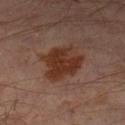follow-up = no biopsy performed (imaged during a skin exam)
tile lighting = cross-polarized
patient = male, aged 63 to 67
size = about 5 mm
acquisition = total-body-photography crop, ~15 mm field of view
automated lesion analysis = a lesion area of about 15 mm² and a shape eccentricity near 0.5; a lesion color around L≈31 a*≈20 b*≈26 in CIELAB and a normalized border contrast of about 10.5; a color-variation rating of about 3/10 and a peripheral color-asymmetry measure near 1; a lesion-detection confidence of about 100/100
anatomic site = the leg